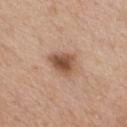Q: Was this lesion biopsied?
A: total-body-photography surveillance lesion; no biopsy
Q: Lesion location?
A: the chest
Q: How large is the lesion?
A: about 3.5 mm
Q: How was this image acquired?
A: ~15 mm crop, total-body skin-cancer survey
Q: What did automated image analysis measure?
A: an area of roughly 7.5 mm², a shape eccentricity near 0.7, and two-axis asymmetry of about 0.3; an average lesion color of about L≈52 a*≈20 b*≈30 (CIELAB), about 13 CIELAB-L* units darker than the surrounding skin, and a normalized border contrast of about 9.5
Q: What are the patient's age and sex?
A: male, roughly 55 years of age
Q: Illumination type?
A: white-light